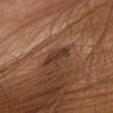Part of a total-body skin-imaging series; this lesion was reviewed on a skin check and was not flagged for biopsy. Approximately 3.5 mm at its widest. From the head or neck. A female subject roughly 50 years of age. An algorithmic analysis of the crop reported a border-irregularity index near 3.5/10, a within-lesion color-variation index near 1.5/10, and a peripheral color-asymmetry measure near 0.5. And it measured a classifier nevus-likeness of about 0/100 and a detector confidence of about 70 out of 100 that the crop contains a lesion. The tile uses cross-polarized illumination. A 15 mm crop from a total-body photograph taken for skin-cancer surveillance.